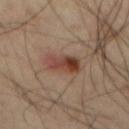The lesion was photographed on a routine skin check and not biopsied; there is no pathology result.
This is a cross-polarized tile.
A male subject, aged around 60.
Longest diameter approximately 4 mm.
On the mid back.
Automated tile analysis of the lesion measured an area of roughly 8 mm², an eccentricity of roughly 0.75, and two-axis asymmetry of about 0.25. It also reported an average lesion color of about L≈40 a*≈21 b*≈26 (CIELAB) and a lesion-to-skin contrast of about 9 (normalized; higher = more distinct).
A 15 mm close-up extracted from a 3D total-body photography capture.